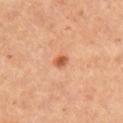  biopsy_status: not biopsied; imaged during a skin examination
  image:
    source: total-body photography crop
    field_of_view_mm: 15
  automated_metrics:
    cielab_L: 58
    cielab_a: 28
    cielab_b: 39
    vs_skin_contrast_norm: 8.0
    color_variation_0_10: 3.5
    nevus_likeness_0_100: 90
    lesion_detection_confidence_0_100: 100
  patient:
    sex: female
    age_approx: 45
  site: right upper arm